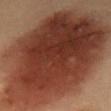The lesion was photographed on a routine skin check and not biopsied; there is no pathology result.
Approximately 16 mm at its widest.
A region of skin cropped from a whole-body photographic capture, roughly 15 mm wide.
The lesion-visualizer software estimated an automated nevus-likeness rating near 100 out of 100 and a detector confidence of about 100 out of 100 that the crop contains a lesion.
From the mid back.
A male patient, in their mid- to late 50s.